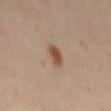Recorded during total-body skin imaging; not selected for excision or biopsy. Located on the left thigh. Longest diameter approximately 3 mm. An algorithmic analysis of the crop reported a mean CIELAB color near L≈50 a*≈19 b*≈31, roughly 11 lightness units darker than nearby skin, and a lesion-to-skin contrast of about 9 (normalized; higher = more distinct). The analysis additionally found border irregularity of about 1.5 on a 0–10 scale, internal color variation of about 2 on a 0–10 scale, and peripheral color asymmetry of about 0.5. A close-up tile cropped from a whole-body skin photograph, about 15 mm across. A female patient, aged approximately 40. The tile uses cross-polarized illumination.